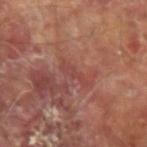notes: no biopsy performed (imaged during a skin exam); patient: male, aged 63 to 67; acquisition: ~15 mm crop, total-body skin-cancer survey; site: the left forearm; lighting: cross-polarized; lesion size: ~3.5 mm (longest diameter).